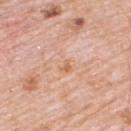Assessment:
Captured during whole-body skin photography for melanoma surveillance; the lesion was not biopsied.
Clinical summary:
A region of skin cropped from a whole-body photographic capture, roughly 15 mm wide. The recorded lesion diameter is about 3 mm. The tile uses white-light illumination. The subject is a male aged 78–82. On the back.